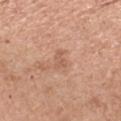– follow-up: catalogued during a skin exam; not biopsied
– patient: female, aged 58 to 62
– size: about 2.5 mm
– imaging modality: total-body-photography crop, ~15 mm field of view
– site: the left forearm
– lighting: white-light
– image-analysis metrics: an area of roughly 3 mm², a shape eccentricity near 0.85, and a symmetry-axis asymmetry near 0.45